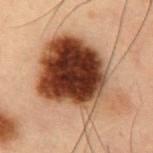Imaged during a routine full-body skin examination; the lesion was not biopsied and no histopathology is available. Cropped from a whole-body photographic skin survey; the tile spans about 15 mm. Located on the front of the torso. The lesion's longest dimension is about 10 mm. A male subject about 55 years old. The tile uses cross-polarized illumination.